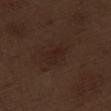No biopsy was performed on this lesion — it was imaged during a full skin examination and was not determined to be concerning. About 3 mm across. Captured under white-light illumination. A male patient aged approximately 70. A lesion tile, about 15 mm wide, cut from a 3D total-body photograph. Located on the left thigh.